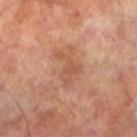Recorded during total-body skin imaging; not selected for excision or biopsy.
The lesion-visualizer software estimated a lesion area of about 6 mm², a shape eccentricity near 0.75, and a symmetry-axis asymmetry near 0.65. The software also gave border irregularity of about 7.5 on a 0–10 scale and a within-lesion color-variation index near 2.5/10. The analysis additionally found a lesion-detection confidence of about 100/100.
The tile uses cross-polarized illumination.
On the left lower leg.
Approximately 4 mm at its widest.
The patient is a male aged 68 to 72.
Cropped from a whole-body photographic skin survey; the tile spans about 15 mm.